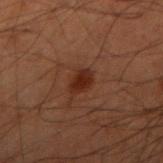Q: Is there a histopathology result?
A: no biopsy performed (imaged during a skin exam)
Q: Patient demographics?
A: male, aged approximately 60
Q: What is the anatomic site?
A: the right upper arm
Q: Automated lesion metrics?
A: a lesion area of about 4.5 mm², an eccentricity of roughly 0.5, and a symmetry-axis asymmetry near 0.2; a lesion–skin lightness drop of about 7; a border-irregularity rating of about 2/10 and a color-variation rating of about 1.5/10; a nevus-likeness score of about 80/100 and lesion-presence confidence of about 100/100
Q: What kind of image is this?
A: ~15 mm tile from a whole-body skin photo
Q: How large is the lesion?
A: ~2.5 mm (longest diameter)